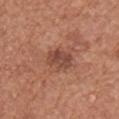Case summary:
- biopsy status: catalogued during a skin exam; not biopsied
- location: the right upper arm
- lesion size: ≈3 mm
- image: ~15 mm tile from a whole-body skin photo
- lighting: white-light illumination
- patient: male, in their mid- to late 70s